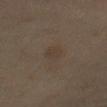workup: imaged on a skin check; not biopsied
location: the abdomen
image source: total-body-photography crop, ~15 mm field of view
subject: male, about 55 years old